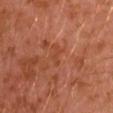Imaged with cross-polarized lighting. Longest diameter approximately 2.5 mm. A male subject, approximately 30 years of age. From the left arm. A close-up tile cropped from a whole-body skin photograph, about 15 mm across. Automated image analysis of the tile measured an eccentricity of roughly 0.9 and a shape-asymmetry score of about 0.6 (0 = symmetric). And it measured a border-irregularity index near 5.5/10, a color-variation rating of about 0/10, and peripheral color asymmetry of about 0. It also reported an automated nevus-likeness rating near 0 out of 100 and lesion-presence confidence of about 100/100.